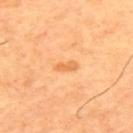Impression:
This lesion was catalogued during total-body skin photography and was not selected for biopsy.
Background:
A male patient in their mid- to late 60s. A region of skin cropped from a whole-body photographic capture, roughly 15 mm wide. Captured under cross-polarized illumination. The lesion is located on the upper back.